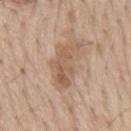notes: imaged on a skin check; not biopsied | lesion diameter: ≈5.5 mm | automated metrics: an average lesion color of about L≈57 a*≈18 b*≈30 (CIELAB), a lesion–skin lightness drop of about 10, and a lesion-to-skin contrast of about 6.5 (normalized; higher = more distinct); border irregularity of about 6.5 on a 0–10 scale, a within-lesion color-variation index near 4.5/10, and a peripheral color-asymmetry measure near 1.5 | lighting: white-light illumination | body site: the back | subject: male, roughly 70 years of age | image source: ~15 mm crop, total-body skin-cancer survey.